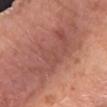The lesion was photographed on a routine skin check and not biopsied; there is no pathology result. The total-body-photography lesion software estimated an outline eccentricity of about 0.85 (0 = round, 1 = elongated) and a shape-asymmetry score of about 0.45 (0 = symmetric). And it measured a lesion color around L≈50 a*≈26 b*≈26 in CIELAB, a lesion–skin lightness drop of about 7, and a normalized border contrast of about 5. The analysis additionally found a nevus-likeness score of about 5/100 and a detector confidence of about 60 out of 100 that the crop contains a lesion. Imaged with white-light lighting. The lesion is on the right forearm. The patient is a female approximately 40 years of age. A 15 mm crop from a total-body photograph taken for skin-cancer surveillance.